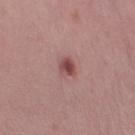biopsy status — no biopsy performed (imaged during a skin exam) | lighting — white-light | subject — female, about 40 years old | image — total-body-photography crop, ~15 mm field of view | body site — the right lower leg.